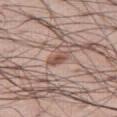Part of a total-body skin-imaging series; this lesion was reviewed on a skin check and was not flagged for biopsy. The lesion's longest dimension is about 3 mm. A region of skin cropped from a whole-body photographic capture, roughly 15 mm wide. A male subject in their mid- to late 50s. From the right thigh.